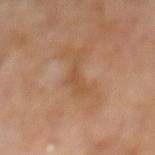Notes:
• notes: catalogued during a skin exam; not biopsied
• anatomic site: the mid back
• patient: male, aged around 70
• imaging modality: 15 mm crop, total-body photography
• lighting: cross-polarized